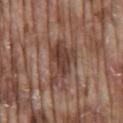| key | value |
|---|---|
| workup | imaged on a skin check; not biopsied |
| patient | male, aged approximately 75 |
| acquisition | ~15 mm tile from a whole-body skin photo |
| lesion size | ≈6 mm |
| location | the mid back |